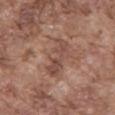Captured during whole-body skin photography for melanoma surveillance; the lesion was not biopsied.
The patient is a male aged 73–77.
A 15 mm close-up extracted from a 3D total-body photography capture.
The lesion is located on the mid back.
About 5 mm across.
This is a white-light tile.